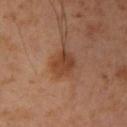The lesion is located on the arm. Automated tile analysis of the lesion measured a lesion color around L≈43 a*≈22 b*≈33 in CIELAB and a lesion–skin lightness drop of about 9. The analysis additionally found a border-irregularity rating of about 1.5/10 and a within-lesion color-variation index near 3.5/10. The analysis additionally found a nevus-likeness score of about 75/100. Cropped from a total-body skin-imaging series; the visible field is about 15 mm. Imaged with cross-polarized lighting. A female subject, aged around 45. The recorded lesion diameter is about 3.5 mm.